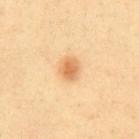Imaged during a routine full-body skin examination; the lesion was not biopsied and no histopathology is available.
The lesion is on the chest.
A close-up tile cropped from a whole-body skin photograph, about 15 mm across.
Imaged with cross-polarized lighting.
A male subject about 40 years old.
Measured at roughly 2.5 mm in maximum diameter.